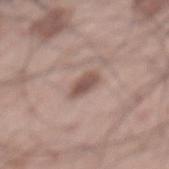Acquisition and patient details: The tile uses white-light illumination. An algorithmic analysis of the crop reported a lesion area of about 4.5 mm², a shape eccentricity near 0.85, and a shape-asymmetry score of about 0.3 (0 = symmetric). The software also gave a border-irregularity rating of about 2.5/10, internal color variation of about 2.5 on a 0–10 scale, and peripheral color asymmetry of about 1. The analysis additionally found a detector confidence of about 100 out of 100 that the crop contains a lesion. On the mid back. A close-up tile cropped from a whole-body skin photograph, about 15 mm across. A male subject, aged 68 to 72. Longest diameter approximately 3 mm.